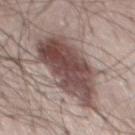Impression: Captured during whole-body skin photography for melanoma surveillance; the lesion was not biopsied.